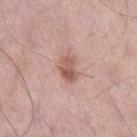Assessment:
Imaged during a routine full-body skin examination; the lesion was not biopsied and no histopathology is available.
Acquisition and patient details:
An algorithmic analysis of the crop reported a symmetry-axis asymmetry near 0.25. It also reported an automated nevus-likeness rating near 70 out of 100 and a lesion-detection confidence of about 100/100. About 3.5 mm across. Cropped from a whole-body photographic skin survey; the tile spans about 15 mm. Imaged with white-light lighting. The lesion is on the leg. A male patient aged approximately 55.